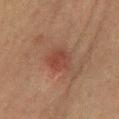This lesion was catalogued during total-body skin photography and was not selected for biopsy. On the mid back. A male patient, aged around 65. The total-body-photography lesion software estimated border irregularity of about 2 on a 0–10 scale and radial color variation of about 1. Measured at roughly 3.5 mm in maximum diameter. A lesion tile, about 15 mm wide, cut from a 3D total-body photograph. This is a cross-polarized tile.